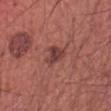Q: Is there a histopathology result?
A: catalogued during a skin exam; not biopsied
Q: What kind of image is this?
A: total-body-photography crop, ~15 mm field of view
Q: Lesion location?
A: the front of the torso
Q: What are the patient's age and sex?
A: male, in their mid-60s